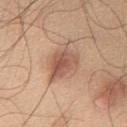Recorded during total-body skin imaging; not selected for excision or biopsy. Measured at roughly 5 mm in maximum diameter. The subject is a male aged 43 to 47. The lesion is on the front of the torso. A roughly 15 mm field-of-view crop from a total-body skin photograph.